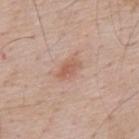Impression:
The lesion was tiled from a total-body skin photograph and was not biopsied.
Image and clinical context:
Approximately 3 mm at its widest. A male patient, aged around 60. This is a white-light tile. A lesion tile, about 15 mm wide, cut from a 3D total-body photograph. On the back. The total-body-photography lesion software estimated an automated nevus-likeness rating near 60 out of 100 and a detector confidence of about 100 out of 100 that the crop contains a lesion.